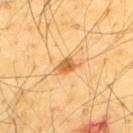workup — total-body-photography surveillance lesion; no biopsy | anatomic site — the upper back | subject — male, aged approximately 65 | diameter — about 3 mm | illumination — cross-polarized illumination | TBP lesion metrics — an area of roughly 4 mm², an outline eccentricity of about 0.8 (0 = round, 1 = elongated), and two-axis asymmetry of about 0.35 | imaging modality — ~15 mm tile from a whole-body skin photo.